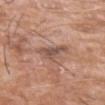Imaged during a routine full-body skin examination; the lesion was not biopsied and no histopathology is available. This image is a 15 mm lesion crop taken from a total-body photograph. Imaged with white-light lighting. Measured at roughly 4 mm in maximum diameter. The lesion is on the arm. The patient is a male roughly 75 years of age.